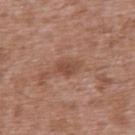biopsy status: no biopsy performed (imaged during a skin exam); patient: male, aged approximately 45; anatomic site: the upper back; lighting: white-light; diameter: ~2.5 mm (longest diameter); image source: ~15 mm crop, total-body skin-cancer survey.